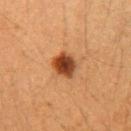This lesion was catalogued during total-body skin photography and was not selected for biopsy.
Measured at roughly 3.5 mm in maximum diameter.
A 15 mm close-up tile from a total-body photography series done for melanoma screening.
A female patient aged around 40.
Located on the right upper arm.